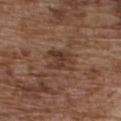- notes — imaged on a skin check; not biopsied
- size — about 3.5 mm
- image — total-body-photography crop, ~15 mm field of view
- illumination — white-light
- patient — male, roughly 75 years of age
- body site — the chest
- automated lesion analysis — a mean CIELAB color near L≈37 a*≈18 b*≈26, roughly 8 lightness units darker than nearby skin, and a normalized lesion–skin contrast near 7; border irregularity of about 3 on a 0–10 scale